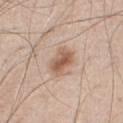Q: Was this lesion biopsied?
A: no biopsy performed (imaged during a skin exam)
Q: How was this image acquired?
A: total-body-photography crop, ~15 mm field of view
Q: Where on the body is the lesion?
A: the chest
Q: Who is the patient?
A: male, aged 43–47